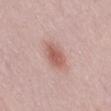<lesion>
  <biopsy_status>not biopsied; imaged during a skin examination</biopsy_status>
  <lesion_size>
    <long_diameter_mm_approx>3.0</long_diameter_mm_approx>
  </lesion_size>
  <patient>
    <sex>female</sex>
    <age_approx>50</age_approx>
  </patient>
  <image>
    <source>total-body photography crop</source>
    <field_of_view_mm>15</field_of_view_mm>
  </image>
  <automated_metrics>
    <border_irregularity_0_10>1.5</border_irregularity_0_10>
    <color_variation_0_10>3.0</color_variation_0_10>
    <peripheral_color_asymmetry>1.0</peripheral_color_asymmetry>
    <nevus_likeness_0_100>95</nevus_likeness_0_100>
    <lesion_detection_confidence_0_100>100</lesion_detection_confidence_0_100>
  </automated_metrics>
  <site>back</site>
</lesion>